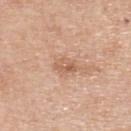Q: Is there a histopathology result?
A: total-body-photography surveillance lesion; no biopsy
Q: What is the imaging modality?
A: total-body-photography crop, ~15 mm field of view
Q: What lighting was used for the tile?
A: white-light
Q: Automated lesion metrics?
A: roughly 9 lightness units darker than nearby skin and a normalized lesion–skin contrast near 6; a border-irregularity rating of about 2.5/10 and a color-variation rating of about 5.5/10; an automated nevus-likeness rating near 0 out of 100 and a lesion-detection confidence of about 100/100
Q: Who is the patient?
A: male, aged 68–72
Q: Where on the body is the lesion?
A: the upper back
Q: How large is the lesion?
A: ≈3 mm